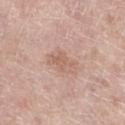{
  "lesion_size": {
    "long_diameter_mm_approx": 3.5
  },
  "lighting": "white-light",
  "site": "left lower leg",
  "patient": {
    "sex": "female",
    "age_approx": 70
  },
  "image": {
    "source": "total-body photography crop",
    "field_of_view_mm": 15
  }
}